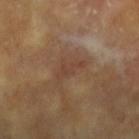* biopsy status: total-body-photography surveillance lesion; no biopsy
* lighting: cross-polarized
* location: the arm
* automated lesion analysis: a lesion–skin lightness drop of about 6; a border-irregularity rating of about 5.5/10
* subject: female, aged 73–77
* acquisition: 15 mm crop, total-body photography
* size: ~3.5 mm (longest diameter)